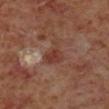Clinical impression: Captured during whole-body skin photography for melanoma surveillance; the lesion was not biopsied. Context: This image is a 15 mm lesion crop taken from a total-body photograph. Imaged with cross-polarized lighting. On the right lower leg. The subject is a male aged around 60.